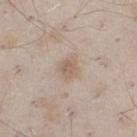Findings:
– biopsy status — catalogued during a skin exam; not biopsied
– patient — male, in their mid-40s
– illumination — white-light
– site — the left thigh
– lesion diameter — ~3 mm (longest diameter)
– image source — ~15 mm crop, total-body skin-cancer survey